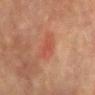Context: The recorded lesion diameter is about 2.5 mm. The lesion is located on the mid back. A male patient aged around 75. Captured under cross-polarized illumination. Cropped from a total-body skin-imaging series; the visible field is about 15 mm.